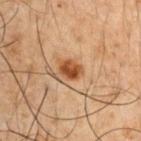{
  "patient": {
    "sex": "male",
    "age_approx": 50
  },
  "image": {
    "source": "total-body photography crop",
    "field_of_view_mm": 15
  },
  "lesion_size": {
    "long_diameter_mm_approx": 3.0
  },
  "lighting": "cross-polarized",
  "site": "left upper arm",
  "automated_metrics": {
    "eccentricity": 0.55,
    "shape_asymmetry": 0.15,
    "cielab_L": 38,
    "cielab_a": 20,
    "cielab_b": 31,
    "vs_skin_darker_L": 11.0,
    "vs_skin_contrast_norm": 10.0,
    "border_irregularity_0_10": 1.5,
    "color_variation_0_10": 4.0,
    "peripheral_color_asymmetry": 1.5,
    "nevus_likeness_0_100": 95,
    "lesion_detection_confidence_0_100": 100
  }
}